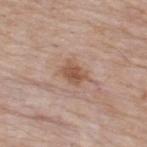Impression: Imaged during a routine full-body skin examination; the lesion was not biopsied and no histopathology is available. Context: A 15 mm crop from a total-body photograph taken for skin-cancer surveillance. The tile uses white-light illumination. The recorded lesion diameter is about 3.5 mm. Located on the upper back. Automated tile analysis of the lesion measured an average lesion color of about L≈54 a*≈19 b*≈29 (CIELAB), about 10 CIELAB-L* units darker than the surrounding skin, and a lesion-to-skin contrast of about 7.5 (normalized; higher = more distinct). The subject is a male in their mid-60s.